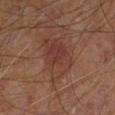body site = the right leg; patient = male, about 60 years old; tile lighting = cross-polarized illumination; lesion size = about 5.5 mm; image source = 15 mm crop, total-body photography.